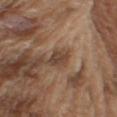The lesion was photographed on a routine skin check and not biopsied; there is no pathology result. A roughly 15 mm field-of-view crop from a total-body skin photograph. A male patient in their mid- to late 70s. On the upper back.